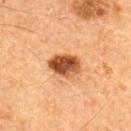No biopsy was performed on this lesion — it was imaged during a full skin examination and was not determined to be concerning. The tile uses cross-polarized illumination. A male subject, aged around 60. A 15 mm close-up extracted from a 3D total-body photography capture. Longest diameter approximately 4 mm.